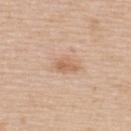Captured during whole-body skin photography for melanoma surveillance; the lesion was not biopsied.
From the upper back.
Captured under white-light illumination.
This image is a 15 mm lesion crop taken from a total-body photograph.
Longest diameter approximately 3 mm.
A female patient, approximately 45 years of age.